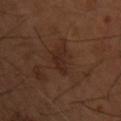Findings:
- location · the chest
- TBP lesion metrics · an area of roughly 5 mm² and a shape eccentricity near 0.75; a mean CIELAB color near L≈24 a*≈17 b*≈22, roughly 5 lightness units darker than nearby skin, and a normalized border contrast of about 6; a border-irregularity index near 4/10, a color-variation rating of about 1.5/10, and peripheral color asymmetry of about 0.5; a nevus-likeness score of about 5/100 and a detector confidence of about 100 out of 100 that the crop contains a lesion
- image source · ~15 mm tile from a whole-body skin photo
- lesion size · ≈3 mm
- patient · male, roughly 55 years of age